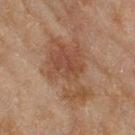The lesion was photographed on a routine skin check and not biopsied; there is no pathology result. Located on the leg. Longest diameter approximately 8 mm. A 15 mm crop from a total-body photograph taken for skin-cancer surveillance. This is a cross-polarized tile. The patient is a female aged 58–62.